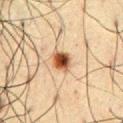notes: imaged on a skin check; not biopsied
image source: 15 mm crop, total-body photography
tile lighting: cross-polarized
subject: male, aged around 60
size: about 2.5 mm
site: the chest